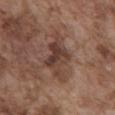notes — no biopsy performed (imaged during a skin exam) | patient — male, aged around 75 | body site — the abdomen | lesion size — ≈6 mm | image — 15 mm crop, total-body photography | tile lighting — white-light.